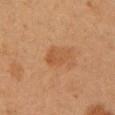notes = imaged on a skin check; not biopsied | location = the left upper arm | imaging modality = ~15 mm tile from a whole-body skin photo | patient = female, aged 38–42.